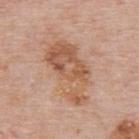No biopsy was performed on this lesion — it was imaged during a full skin examination and was not determined to be concerning. A male patient aged around 75. The tile uses white-light illumination. The lesion is on the upper back. The recorded lesion diameter is about 7.5 mm. This image is a 15 mm lesion crop taken from a total-body photograph.